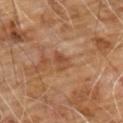lesion diameter: about 2.5 mm | acquisition: total-body-photography crop, ~15 mm field of view | location: the chest | illumination: cross-polarized | subject: male, in their 60s | image-analysis metrics: a lesion area of about 3.5 mm², an outline eccentricity of about 0.6 (0 = round, 1 = elongated), and a shape-asymmetry score of about 0.35 (0 = symmetric); a border-irregularity index near 3/10, a within-lesion color-variation index near 4/10, and radial color variation of about 1.5.